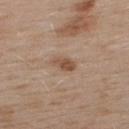The lesion was tiled from a total-body skin photograph and was not biopsied.
The lesion is on the upper back.
A 15 mm close-up tile from a total-body photography series done for melanoma screening.
The lesion's longest dimension is about 3 mm.
Imaged with white-light lighting.
The patient is a male aged 53–57.